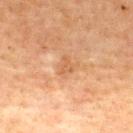Part of a total-body skin-imaging series; this lesion was reviewed on a skin check and was not flagged for biopsy.